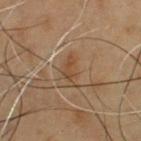{
  "site": "front of the torso",
  "patient": {
    "sex": "male",
    "age_approx": 55
  },
  "image": {
    "source": "total-body photography crop",
    "field_of_view_mm": 15
  }
}